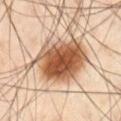biopsy status: catalogued during a skin exam; not biopsied
image: total-body-photography crop, ~15 mm field of view
location: the leg
subject: male, in their mid- to late 50s